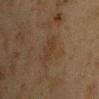Context:
The lesion is on the left upper arm. An algorithmic analysis of the crop reported a shape eccentricity near 0.9. The software also gave an automated nevus-likeness rating near 0 out of 100 and a detector confidence of about 100 out of 100 that the crop contains a lesion. A male patient, aged 43 to 47. A lesion tile, about 15 mm wide, cut from a 3D total-body photograph. About 4 mm across. Imaged with cross-polarized lighting.